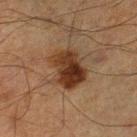Assessment:
The lesion was tiled from a total-body skin photograph and was not biopsied.
Acquisition and patient details:
A male subject aged 63–67. On the right lower leg. A lesion tile, about 15 mm wide, cut from a 3D total-body photograph. The lesion's longest dimension is about 4.5 mm.